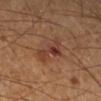biopsy_status: not biopsied; imaged during a skin examination
automated_metrics:
  cielab_L: 37
  cielab_a: 22
  cielab_b: 27
  vs_skin_darker_L: 8.0
  vs_skin_contrast_norm: 7.5
  border_irregularity_0_10: 4.5
  peripheral_color_asymmetry: 2.0
  lesion_detection_confidence_0_100: 100
patient:
  sex: male
  age_approx: 60
site: right lower leg
image:
  source: total-body photography crop
  field_of_view_mm: 15
lesion_size:
  long_diameter_mm_approx: 4.5
lighting: cross-polarized